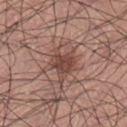Assessment: This lesion was catalogued during total-body skin photography and was not selected for biopsy. Acquisition and patient details: About 3 mm across. A male patient, about 25 years old. Cropped from a whole-body photographic skin survey; the tile spans about 15 mm. Captured under white-light illumination. From the right lower leg. Automated image analysis of the tile measured an average lesion color of about L≈43 a*≈22 b*≈23 (CIELAB), a lesion–skin lightness drop of about 11, and a normalized lesion–skin contrast near 8.5. And it measured a border-irregularity rating of about 2.5/10, a color-variation rating of about 3/10, and peripheral color asymmetry of about 1.